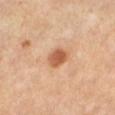Q: Was this lesion biopsied?
A: no biopsy performed (imaged during a skin exam)
Q: Lesion location?
A: the left lower leg
Q: What kind of image is this?
A: ~15 mm tile from a whole-body skin photo
Q: Patient demographics?
A: female, approximately 45 years of age
Q: What lighting was used for the tile?
A: cross-polarized